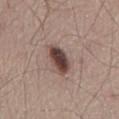Captured during whole-body skin photography for melanoma surveillance; the lesion was not biopsied.
From the front of the torso.
Approximately 4 mm at its widest.
A male subject aged 58 to 62.
Cropped from a whole-body photographic skin survey; the tile spans about 15 mm.
This is a white-light tile.